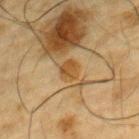Findings:
- workup: no biopsy performed (imaged during a skin exam)
- illumination: cross-polarized
- imaging modality: ~15 mm tile from a whole-body skin photo
- location: the chest
- subject: male, about 85 years old or older
- diameter: ≈2.5 mm